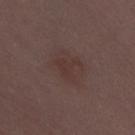The lesion was tiled from a total-body skin photograph and was not biopsied. The lesion-visualizer software estimated a lesion-detection confidence of about 100/100. Imaged with white-light lighting. The subject is a female about 30 years old. This image is a 15 mm lesion crop taken from a total-body photograph. The lesion is on the lower back.